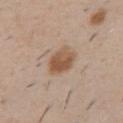Clinical impression:
No biopsy was performed on this lesion — it was imaged during a full skin examination and was not determined to be concerning.
Acquisition and patient details:
This is a white-light tile. The lesion is on the chest. This image is a 15 mm lesion crop taken from a total-body photograph. Approximately 4 mm at its widest. A male patient approximately 50 years of age.